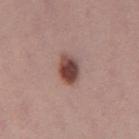Assessment: Recorded during total-body skin imaging; not selected for excision or biopsy. Context: On the left thigh. A female patient in their 30s. A lesion tile, about 15 mm wide, cut from a 3D total-body photograph. Approximately 3.5 mm at its widest.